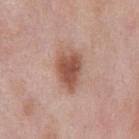Impression: This lesion was catalogued during total-body skin photography and was not selected for biopsy. Clinical summary: A male subject, roughly 55 years of age. A close-up tile cropped from a whole-body skin photograph, about 15 mm across. The lesion-visualizer software estimated an area of roughly 11 mm². The software also gave a normalized border contrast of about 9.5. The analysis additionally found a border-irregularity rating of about 3/10 and a color-variation rating of about 3/10. And it measured a classifier nevus-likeness of about 95/100 and a lesion-detection confidence of about 100/100. Longest diameter approximately 4.5 mm. Imaged with white-light lighting. On the front of the torso.